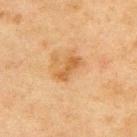patient = male, approximately 45 years of age; site = the back; image source = ~15 mm tile from a whole-body skin photo; lighting = cross-polarized illumination; lesion size = ~3 mm (longest diameter).